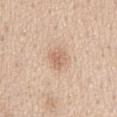Recorded during total-body skin imaging; not selected for excision or biopsy. A region of skin cropped from a whole-body photographic capture, roughly 15 mm wide. The total-body-photography lesion software estimated a lesion color around L≈65 a*≈18 b*≈31 in CIELAB, roughly 9 lightness units darker than nearby skin, and a lesion-to-skin contrast of about 6 (normalized; higher = more distinct). It also reported a nevus-likeness score of about 30/100 and a lesion-detection confidence of about 100/100. About 3 mm across. A male patient in their 60s. The lesion is on the mid back. This is a white-light tile.